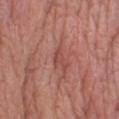  lesion_size:
    long_diameter_mm_approx: 2.5
  lighting: white-light
  automated_metrics:
    area_mm2_approx: 2.5
    eccentricity: 0.85
    shape_asymmetry: 0.65
    nevus_likeness_0_100: 0
    lesion_detection_confidence_0_100: 55
  image:
    source: total-body photography crop
    field_of_view_mm: 15
  patient:
    sex: female
    age_approx: 55
  site: right lower leg